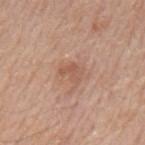The lesion is on the mid back.
The subject is a male roughly 80 years of age.
Captured under white-light illumination.
Cropped from a total-body skin-imaging series; the visible field is about 15 mm.
The lesion-visualizer software estimated an area of roughly 4.5 mm², a shape eccentricity near 0.65, and two-axis asymmetry of about 0.5. The analysis additionally found a lesion–skin lightness drop of about 7. And it measured a nevus-likeness score of about 5/100 and lesion-presence confidence of about 100/100.
Longest diameter approximately 2.5 mm.